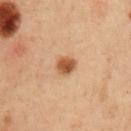Notes:
* subject: male, approximately 60 years of age
* location: the arm
* image: ~15 mm crop, total-body skin-cancer survey
* size: about 2.5 mm